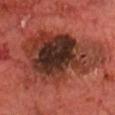notes=total-body-photography surveillance lesion; no biopsy | location=the head or neck | diameter=about 11 mm | image source=~15 mm tile from a whole-body skin photo | patient=male, approximately 70 years of age | image-analysis metrics=a mean CIELAB color near L≈34 a*≈25 b*≈27, about 13 CIELAB-L* units darker than the surrounding skin, and a normalized lesion–skin contrast near 10.5; a border-irregularity index near 5.5/10 and internal color variation of about 8.5 on a 0–10 scale; a detector confidence of about 100 out of 100 that the crop contains a lesion | illumination=cross-polarized.